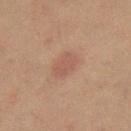Case summary:
* notes — imaged on a skin check; not biopsied